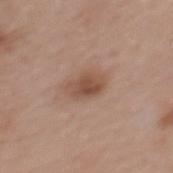This lesion was catalogued during total-body skin photography and was not selected for biopsy. The tile uses white-light illumination. A region of skin cropped from a whole-body photographic capture, roughly 15 mm wide. The patient is a female in their 60s. Longest diameter approximately 3.5 mm. The lesion is located on the upper back.